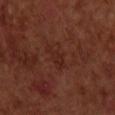{
  "biopsy_status": "not biopsied; imaged during a skin examination",
  "image": {
    "source": "total-body photography crop",
    "field_of_view_mm": 15
  },
  "site": "chest",
  "lighting": "cross-polarized",
  "patient": {
    "sex": "male",
    "age_approx": 70
  },
  "lesion_size": {
    "long_diameter_mm_approx": 3.0
  }
}